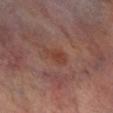biopsy status = catalogued during a skin exam; not biopsied.